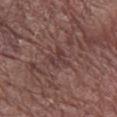{
  "biopsy_status": "not biopsied; imaged during a skin examination",
  "patient": {
    "sex": "male",
    "age_approx": 65
  },
  "site": "left forearm",
  "lesion_size": {
    "long_diameter_mm_approx": 2.5
  },
  "image": {
    "source": "total-body photography crop",
    "field_of_view_mm": 15
  },
  "lighting": "white-light",
  "automated_metrics": {
    "area_mm2_approx": 4.0,
    "eccentricity": 0.55,
    "shape_asymmetry": 0.55,
    "cielab_L": 37,
    "cielab_a": 20,
    "cielab_b": 19,
    "vs_skin_darker_L": 6.0,
    "vs_skin_contrast_norm": 6.0,
    "nevus_likeness_0_100": 0
  }
}